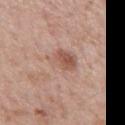lesion size: about 5 mm | tile lighting: white-light | location: the mid back | image source: ~15 mm tile from a whole-body skin photo | patient: male, approximately 65 years of age.